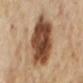* workup — no biopsy performed (imaged during a skin exam)
* patient — female, aged 53–57
* anatomic site — the mid back
* imaging modality — ~15 mm crop, total-body skin-cancer survey
* TBP lesion metrics — a border-irregularity rating of about 2/10, a within-lesion color-variation index near 7/10, and radial color variation of about 2.5; a nevus-likeness score of about 65/100 and a detector confidence of about 100 out of 100 that the crop contains a lesion